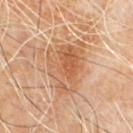Approximately 6 mm at its widest.
Imaged with cross-polarized lighting.
Cropped from a whole-body photographic skin survey; the tile spans about 15 mm.
The lesion is located on the upper back.
Automated image analysis of the tile measured a lesion area of about 20 mm², an eccentricity of roughly 0.7, and a shape-asymmetry score of about 0.35 (0 = symmetric). The software also gave an automated nevus-likeness rating near 40 out of 100 and a lesion-detection confidence of about 100/100.
The subject is a male in their 60s.